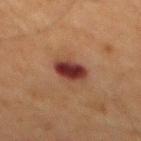Clinical impression: No biopsy was performed on this lesion — it was imaged during a full skin examination and was not determined to be concerning. Acquisition and patient details: The lesion-visualizer software estimated a within-lesion color-variation index near 5/10 and radial color variation of about 1.5. Longest diameter approximately 3.5 mm. Cropped from a total-body skin-imaging series; the visible field is about 15 mm. The lesion is located on the mid back. A male patient, approximately 65 years of age.